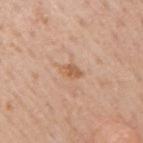Clinical impression:
Imaged during a routine full-body skin examination; the lesion was not biopsied and no histopathology is available.
Image and clinical context:
Captured under white-light illumination. A 15 mm close-up extracted from a 3D total-body photography capture. A male subject, roughly 75 years of age. The lesion is located on the right upper arm. Longest diameter approximately 2.5 mm. The lesion-visualizer software estimated a footprint of about 3 mm² and a shape eccentricity near 0.85. The software also gave border irregularity of about 2.5 on a 0–10 scale, a color-variation rating of about 1.5/10, and peripheral color asymmetry of about 0.5.